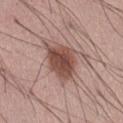Impression: The lesion was photographed on a routine skin check and not biopsied; there is no pathology result. Acquisition and patient details: Captured under white-light illumination. The subject is a male about 55 years old. Located on the abdomen. This image is a 15 mm lesion crop taken from a total-body photograph. Measured at roughly 5 mm in maximum diameter.